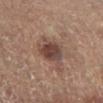<case>
  <biopsy_status>not biopsied; imaged during a skin examination</biopsy_status>
  <site>left lower leg</site>
  <automated_metrics>
    <eccentricity>0.55</eccentricity>
    <shape_asymmetry>0.3</shape_asymmetry>
    <vs_skin_darker_L>11.0</vs_skin_darker_L>
    <vs_skin_contrast_norm>9.0</vs_skin_contrast_norm>
    <nevus_likeness_0_100>75</nevus_likeness_0_100>
    <lesion_detection_confidence_0_100>100</lesion_detection_confidence_0_100>
  </automated_metrics>
  <patient>
    <sex>male</sex>
    <age_approx>65</age_approx>
  </patient>
  <image>
    <source>total-body photography crop</source>
    <field_of_view_mm>15</field_of_view_mm>
  </image>
  <lesion_size>
    <long_diameter_mm_approx>4.0</long_diameter_mm_approx>
  </lesion_size>
</case>